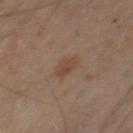No biopsy was performed on this lesion — it was imaged during a full skin examination and was not determined to be concerning. The subject is a male in their mid-60s. From the chest. A roughly 15 mm field-of-view crop from a total-body skin photograph. The lesion's longest dimension is about 3 mm. The tile uses cross-polarized illumination. An algorithmic analysis of the crop reported a lesion area of about 4 mm². The analysis additionally found a mean CIELAB color near L≈43 a*≈17 b*≈28, a lesion–skin lightness drop of about 6, and a normalized border contrast of about 6. The software also gave border irregularity of about 1.5 on a 0–10 scale and a peripheral color-asymmetry measure near 0.5.